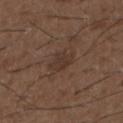| field | value |
|---|---|
| follow-up | total-body-photography surveillance lesion; no biopsy |
| acquisition | ~15 mm tile from a whole-body skin photo |
| site | the upper back |
| patient | male, in their 50s |